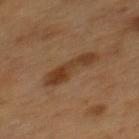| key | value |
|---|---|
| biopsy status | catalogued during a skin exam; not biopsied |
| patient | male, aged 53 to 57 |
| imaging modality | ~15 mm tile from a whole-body skin photo |
| body site | the mid back |
| image-analysis metrics | a lesion color around L≈34 a*≈18 b*≈29 in CIELAB, a lesion–skin lightness drop of about 9, and a normalized lesion–skin contrast near 8; a classifier nevus-likeness of about 45/100 |
| size | about 6 mm |
| tile lighting | cross-polarized |